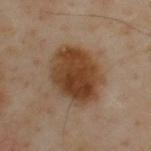Impression: Part of a total-body skin-imaging series; this lesion was reviewed on a skin check and was not flagged for biopsy. Context: Automated tile analysis of the lesion measured a mean CIELAB color near L≈41 a*≈19 b*≈31 and roughly 13 lightness units darker than nearby skin. The software also gave border irregularity of about 1.5 on a 0–10 scale, internal color variation of about 6 on a 0–10 scale, and radial color variation of about 2. The software also gave an automated nevus-likeness rating near 100 out of 100 and lesion-presence confidence of about 100/100. A male patient aged 53–57. A lesion tile, about 15 mm wide, cut from a 3D total-body photograph. Approximately 6.5 mm at its widest. Imaged with cross-polarized lighting. Located on the back.